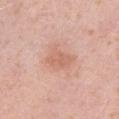This lesion was catalogued during total-body skin photography and was not selected for biopsy. A 15 mm crop from a total-body photograph taken for skin-cancer surveillance. The lesion is located on the leg. Captured under white-light illumination. A female patient, in their 40s.